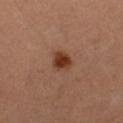Notes:
– size — about 3 mm
– tile lighting — cross-polarized illumination
– image-analysis metrics — an area of roughly 5 mm² and an outline eccentricity of about 0.65 (0 = round, 1 = elongated); a mean CIELAB color near L≈37 a*≈24 b*≈31, roughly 12 lightness units darker than nearby skin, and a lesion-to-skin contrast of about 10.5 (normalized; higher = more distinct); an automated nevus-likeness rating near 100 out of 100 and lesion-presence confidence of about 100/100
– patient — female, aged 28 to 32
– imaging modality — ~15 mm crop, total-body skin-cancer survey
– anatomic site — the right thigh